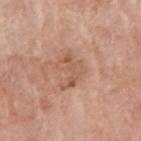<lesion>
<biopsy_status>not biopsied; imaged during a skin examination</biopsy_status>
<patient>
  <sex>male</sex>
  <age_approx>60</age_approx>
</patient>
<lighting>white-light</lighting>
<site>right upper arm</site>
<lesion_size>
  <long_diameter_mm_approx>4.5</long_diameter_mm_approx>
</lesion_size>
<image>
  <source>total-body photography crop</source>
  <field_of_view_mm>15</field_of_view_mm>
</image>
</lesion>